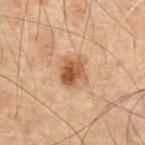This lesion was catalogued during total-body skin photography and was not selected for biopsy. An algorithmic analysis of the crop reported internal color variation of about 4.5 on a 0–10 scale and peripheral color asymmetry of about 2. A male patient, aged 68–72. The tile uses cross-polarized illumination. From the abdomen. A 15 mm close-up tile from a total-body photography series done for melanoma screening. The lesion's longest dimension is about 3.5 mm.